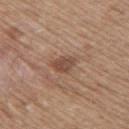{
  "biopsy_status": "not biopsied; imaged during a skin examination",
  "automated_metrics": {
    "cielab_L": 47,
    "cielab_a": 19,
    "cielab_b": 27,
    "vs_skin_darker_L": 10.0,
    "vs_skin_contrast_norm": 7.5,
    "border_irregularity_0_10": 3.0,
    "color_variation_0_10": 3.0,
    "peripheral_color_asymmetry": 1.0,
    "nevus_likeness_0_100": 0,
    "lesion_detection_confidence_0_100": 100
  },
  "image": {
    "source": "total-body photography crop",
    "field_of_view_mm": 15
  },
  "site": "back",
  "patient": {
    "sex": "female",
    "age_approx": 60
  },
  "lesion_size": {
    "long_diameter_mm_approx": 3.0
  },
  "lighting": "white-light"
}